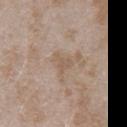Clinical impression: Imaged during a routine full-body skin examination; the lesion was not biopsied and no histopathology is available.